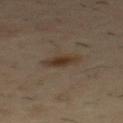No biopsy was performed on this lesion — it was imaged during a full skin examination and was not determined to be concerning. Longest diameter approximately 3 mm. From the mid back. A male patient, in their mid-50s. A roughly 15 mm field-of-view crop from a total-body skin photograph. Automated image analysis of the tile measured an area of roughly 5 mm² and a shape eccentricity near 0.8. And it measured a lesion–skin lightness drop of about 8. And it measured a border-irregularity rating of about 2/10, internal color variation of about 2.5 on a 0–10 scale, and a peripheral color-asymmetry measure near 1.